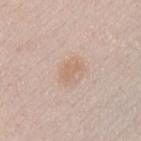The lesion was tiled from a total-body skin photograph and was not biopsied.
Located on the chest.
A male patient, approximately 40 years of age.
Cropped from a total-body skin-imaging series; the visible field is about 15 mm.
About 2.5 mm across.
The lesion-visualizer software estimated a lesion area of about 3 mm², an outline eccentricity of about 0.8 (0 = round, 1 = elongated), and two-axis asymmetry of about 0.35. It also reported a mean CIELAB color near L≈64 a*≈18 b*≈29, a lesion–skin lightness drop of about 6, and a lesion-to-skin contrast of about 5 (normalized; higher = more distinct).